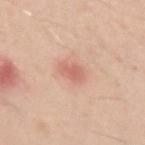Case summary:
• biopsy status · imaged on a skin check; not biopsied
• image · ~15 mm crop, total-body skin-cancer survey
• image-analysis metrics · a border-irregularity rating of about 2.5/10, a color-variation rating of about 1.5/10, and peripheral color asymmetry of about 0.5
• lesion size · ≈3 mm
• subject · male, aged approximately 30
• body site · the left upper arm